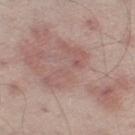No biopsy was performed on this lesion — it was imaged during a full skin examination and was not determined to be concerning.
Approximately 14.5 mm at its widest.
A male patient in their mid- to late 60s.
On the right thigh.
Cropped from a total-body skin-imaging series; the visible field is about 15 mm.
Captured under white-light illumination.